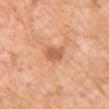{"image": {"source": "total-body photography crop", "field_of_view_mm": 15}, "patient": {"sex": "female", "age_approx": 40}, "site": "left upper arm"}